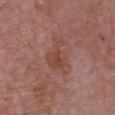No biopsy was performed on this lesion — it was imaged during a full skin examination and was not determined to be concerning. The lesion is located on the chest. An algorithmic analysis of the crop reported a lesion–skin lightness drop of about 7 and a normalized lesion–skin contrast near 6.5. The analysis additionally found border irregularity of about 5.5 on a 0–10 scale, internal color variation of about 3 on a 0–10 scale, and a peripheral color-asymmetry measure near 1. And it measured a nevus-likeness score of about 0/100 and a lesion-detection confidence of about 100/100. This is a white-light tile. A lesion tile, about 15 mm wide, cut from a 3D total-body photograph. A male subject aged 83 or older.